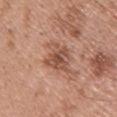Findings:
– site — the back
– image — total-body-photography crop, ~15 mm field of view
– subject — male, aged 48 to 52